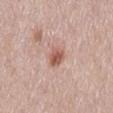notes = imaged on a skin check; not biopsied | imaging modality = total-body-photography crop, ~15 mm field of view | site = the mid back | patient = male, aged 53–57 | automated lesion analysis = an average lesion color of about L≈57 a*≈23 b*≈27 (CIELAB), roughly 12 lightness units darker than nearby skin, and a normalized border contrast of about 8; an automated nevus-likeness rating near 95 out of 100.